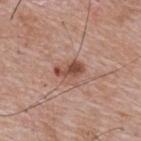  lighting: white-light
  image:
    source: total-body photography crop
    field_of_view_mm: 15
  site: upper back
  patient:
    sex: male
    age_approx: 65
  lesion_size:
    long_diameter_mm_approx: 3.5
  automated_metrics:
    area_mm2_approx: 5.5
    eccentricity: 0.85
    shape_asymmetry: 0.2
    cielab_L: 49
    cielab_a: 23
    cielab_b: 27
    border_irregularity_0_10: 2.5
    color_variation_0_10: 4.5
    peripheral_color_asymmetry: 1.5